A roughly 15 mm field-of-view crop from a total-body skin photograph; the lesion is on the back; a male patient, aged approximately 55 — 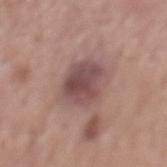The recorded lesion diameter is about 4.5 mm.
Captured under white-light illumination.
The biopsy diagnosis was a dysplastic (Clark) nevus.